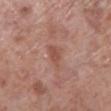notes: catalogued during a skin exam; not biopsied | acquisition: total-body-photography crop, ~15 mm field of view | patient: male, aged approximately 70 | tile lighting: white-light illumination | TBP lesion metrics: a lesion area of about 5 mm², a shape eccentricity near 0.8, and a shape-asymmetry score of about 0.35 (0 = symmetric); a nevus-likeness score of about 0/100 | lesion diameter: ≈3 mm | location: the leg.